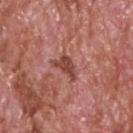Q: Was a biopsy performed?
A: imaged on a skin check; not biopsied
Q: What are the patient's age and sex?
A: male, aged around 60
Q: How was this image acquired?
A: ~15 mm tile from a whole-body skin photo
Q: Where on the body is the lesion?
A: the head or neck